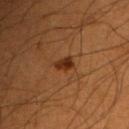Part of a total-body skin-imaging series; this lesion was reviewed on a skin check and was not flagged for biopsy. The lesion is on the arm. The patient is a male aged around 50. This image is a 15 mm lesion crop taken from a total-body photograph.